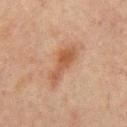follow-up: no biopsy performed (imaged during a skin exam) | tile lighting: cross-polarized illumination | site: the mid back | lesion size: ≈5.5 mm | imaging modality: 15 mm crop, total-body photography | subject: male, aged 63 to 67 | TBP lesion metrics: an area of roughly 8 mm², a shape eccentricity near 0.95, and a shape-asymmetry score of about 0.35 (0 = symmetric); an automated nevus-likeness rating near 95 out of 100.